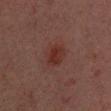| feature | finding |
|---|---|
| follow-up | catalogued during a skin exam; not biopsied |
| image source | 15 mm crop, total-body photography |
| patient | male, aged 28 to 32 |
| site | the chest |